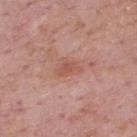| feature | finding |
|---|---|
| notes | total-body-photography surveillance lesion; no biopsy |